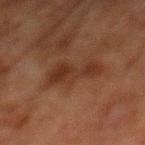Imaged during a routine full-body skin examination; the lesion was not biopsied and no histopathology is available. A close-up tile cropped from a whole-body skin photograph, about 15 mm across. Measured at roughly 5 mm in maximum diameter. The subject is a male aged approximately 80. Automated tile analysis of the lesion measured a lesion area of about 11 mm² and an eccentricity of roughly 0.85. The analysis additionally found a mean CIELAB color near L≈26 a*≈17 b*≈24 and about 6 CIELAB-L* units darker than the surrounding skin. The analysis additionally found a border-irregularity index near 5/10. The software also gave a classifier nevus-likeness of about 0/100 and a detector confidence of about 100 out of 100 that the crop contains a lesion. From the back.